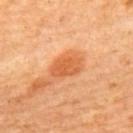<record>
  <biopsy_status>not biopsied; imaged during a skin examination</biopsy_status>
  <patient>
    <sex>female</sex>
    <age_approx>65</age_approx>
  </patient>
  <site>upper back</site>
  <image>
    <source>total-body photography crop</source>
    <field_of_view_mm>15</field_of_view_mm>
  </image>
</record>